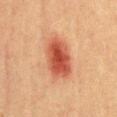- notes · catalogued during a skin exam; not biopsied
- size · ≈6 mm
- site · the chest
- tile lighting · cross-polarized illumination
- acquisition · total-body-photography crop, ~15 mm field of view
- subject · male, aged 38 to 42
- automated lesion analysis · an area of roughly 14 mm² and a symmetry-axis asymmetry near 0.15; a border-irregularity index near 2/10, internal color variation of about 5 on a 0–10 scale, and a peripheral color-asymmetry measure near 1.5